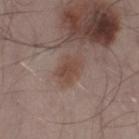  lesion_size:
    long_diameter_mm_approx: 3.5
  lighting: white-light
  image:
    source: total-body photography crop
    field_of_view_mm: 15
  patient:
    sex: male
    age_approx: 55
  automated_metrics:
    eccentricity: 0.65
    cielab_L: 45
    cielab_a: 17
    cielab_b: 23
    vs_skin_darker_L: 7.0
    vs_skin_contrast_norm: 6.5
    nevus_likeness_0_100: 15
  site: left thigh